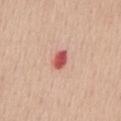| feature | finding |
|---|---|
| biopsy status | catalogued during a skin exam; not biopsied |
| patient | female, aged around 50 |
| location | the back |
| image | ~15 mm crop, total-body skin-cancer survey |